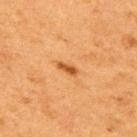{
  "biopsy_status": "not biopsied; imaged during a skin examination",
  "lighting": "cross-polarized",
  "image": {
    "source": "total-body photography crop",
    "field_of_view_mm": 15
  },
  "site": "right upper arm",
  "patient": {
    "sex": "female",
    "age_approx": 40
  },
  "lesion_size": {
    "long_diameter_mm_approx": 2.5
  }
}